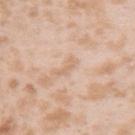image: ~15 mm tile from a whole-body skin photo | body site: the right upper arm | automated metrics: an area of roughly 2 mm², a shape eccentricity near 0.9, and a symmetry-axis asymmetry near 0.5 | illumination: white-light illumination | subject: female, in their mid- to late 20s.